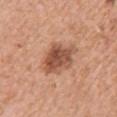The lesion was tiled from a total-body skin photograph and was not biopsied.
Located on the right upper arm.
A female patient, aged 38–42.
This image is a 15 mm lesion crop taken from a total-body photograph.